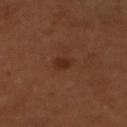Impression: No biopsy was performed on this lesion — it was imaged during a full skin examination and was not determined to be concerning. Background: This is a cross-polarized tile. The lesion-visualizer software estimated an area of roughly 3 mm² and an outline eccentricity of about 0.6 (0 = round, 1 = elongated). It also reported a lesion color around L≈29 a*≈22 b*≈28 in CIELAB. The software also gave a border-irregularity rating of about 2/10 and a peripheral color-asymmetry measure near 0.5. A female subject aged 53 to 57. Located on the left lower leg. Cropped from a total-body skin-imaging series; the visible field is about 15 mm.